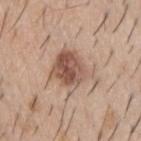Q: Was this lesion biopsied?
A: total-body-photography surveillance lesion; no biopsy
Q: Illumination type?
A: white-light illumination
Q: What are the patient's age and sex?
A: male, aged around 60
Q: Automated lesion metrics?
A: a mean CIELAB color near L≈54 a*≈19 b*≈27 and a lesion–skin lightness drop of about 13; a border-irregularity index near 4.5/10, a color-variation rating of about 7/10, and a peripheral color-asymmetry measure near 2.5; an automated nevus-likeness rating near 45 out of 100 and a detector confidence of about 100 out of 100 that the crop contains a lesion
Q: Where on the body is the lesion?
A: the upper back
Q: What is the imaging modality?
A: ~15 mm crop, total-body skin-cancer survey